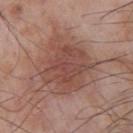biopsy status — total-body-photography surveillance lesion; no biopsy | site — the chest | diameter — about 7 mm | illumination — white-light illumination | subject — male, roughly 65 years of age | imaging modality — ~15 mm tile from a whole-body skin photo.